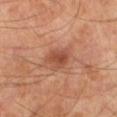Q: What kind of image is this?
A: total-body-photography crop, ~15 mm field of view
Q: Who is the patient?
A: male, in their 70s
Q: What is the anatomic site?
A: the left lower leg
Q: Illumination type?
A: cross-polarized illumination